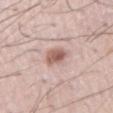biopsy status — imaged on a skin check; not biopsied
image source — total-body-photography crop, ~15 mm field of view
subject — male, in their mid-50s
size — ~3 mm (longest diameter)
TBP lesion metrics — an average lesion color of about L≈59 a*≈20 b*≈24 (CIELAB), a lesion–skin lightness drop of about 13, and a normalized border contrast of about 8.5; internal color variation of about 4 on a 0–10 scale and radial color variation of about 1.5